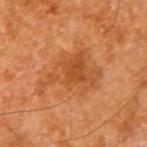Recorded during total-body skin imaging; not selected for excision or biopsy.